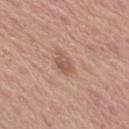The lesion is located on the arm.
The recorded lesion diameter is about 3 mm.
Automated tile analysis of the lesion measured an area of roughly 4 mm² and a shape eccentricity near 0.85. The analysis additionally found a mean CIELAB color near L≈55 a*≈20 b*≈26. And it measured a border-irregularity index near 2.5/10 and a within-lesion color-variation index near 3/10. The software also gave a nevus-likeness score of about 5/100 and a detector confidence of about 100 out of 100 that the crop contains a lesion.
A 15 mm crop from a total-body photograph taken for skin-cancer surveillance.
A female subject aged 63 to 67.